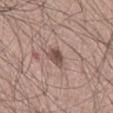Recorded during total-body skin imaging; not selected for excision or biopsy.
Located on the front of the torso.
A lesion tile, about 15 mm wide, cut from a 3D total-body photograph.
Approximately 2.5 mm at its widest.
Captured under white-light illumination.
Automated image analysis of the tile measured an area of roughly 4.5 mm², an outline eccentricity of about 0.65 (0 = round, 1 = elongated), and a shape-asymmetry score of about 0.2 (0 = symmetric). The software also gave a classifier nevus-likeness of about 35/100 and lesion-presence confidence of about 100/100.
A male patient, roughly 65 years of age.